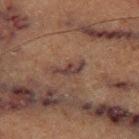Q: Was a biopsy performed?
A: catalogued during a skin exam; not biopsied
Q: Where on the body is the lesion?
A: the leg
Q: What is the imaging modality?
A: 15 mm crop, total-body photography
Q: Patient demographics?
A: male, aged around 75